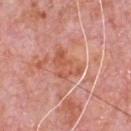notes: imaged on a skin check; not biopsied
size: ≈4 mm
acquisition: ~15 mm crop, total-body skin-cancer survey
image-analysis metrics: a mean CIELAB color near L≈57 a*≈29 b*≈33, about 8 CIELAB-L* units darker than the surrounding skin, and a lesion-to-skin contrast of about 6.5 (normalized; higher = more distinct); a nevus-likeness score of about 0/100 and a lesion-detection confidence of about 95/100
subject: male, roughly 80 years of age
anatomic site: the chest